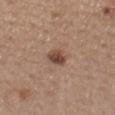| key | value |
|---|---|
| workup | total-body-photography surveillance lesion; no biopsy |
| subject | female, aged approximately 65 |
| acquisition | ~15 mm tile from a whole-body skin photo |
| location | the mid back |
| diameter | ≈2.5 mm |